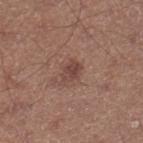* biopsy status: no biopsy performed (imaged during a skin exam)
* patient: male, aged 63–67
* size: about 2.5 mm
* lighting: white-light illumination
* site: the right lower leg
* TBP lesion metrics: a lesion area of about 3.5 mm²; a mean CIELAB color near L≈43 a*≈20 b*≈24, roughly 9 lightness units darker than nearby skin, and a lesion-to-skin contrast of about 7 (normalized; higher = more distinct); a border-irregularity rating of about 2.5/10, internal color variation of about 2.5 on a 0–10 scale, and peripheral color asymmetry of about 1
* imaging modality: total-body-photography crop, ~15 mm field of view